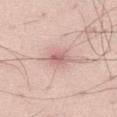Q: Is there a histopathology result?
A: total-body-photography surveillance lesion; no biopsy
Q: What are the patient's age and sex?
A: male, aged around 35
Q: How was this image acquired?
A: ~15 mm tile from a whole-body skin photo
Q: Where on the body is the lesion?
A: the left thigh